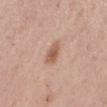Assessment: Recorded during total-body skin imaging; not selected for excision or biopsy. Clinical summary: Approximately 3 mm at its widest. This is a white-light tile. A 15 mm crop from a total-body photograph taken for skin-cancer surveillance. Located on the left forearm. The patient is a female aged 48–52.